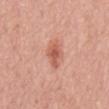Impression: No biopsy was performed on this lesion — it was imaged during a full skin examination and was not determined to be concerning. Image and clinical context: A 15 mm crop from a total-body photograph taken for skin-cancer surveillance. A male subject, about 65 years old. From the mid back.